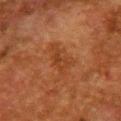This is a cross-polarized tile. Cropped from a total-body skin-imaging series; the visible field is about 15 mm. The lesion's longest dimension is about 4.5 mm. The lesion is located on the chest. The subject is a female in their 50s.